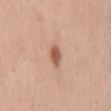Captured during whole-body skin photography for melanoma surveillance; the lesion was not biopsied. The total-body-photography lesion software estimated a footprint of about 4 mm², a shape eccentricity near 0.8, and a shape-asymmetry score of about 0.3 (0 = symmetric). The software also gave a mean CIELAB color near L≈57 a*≈23 b*≈31 and a normalized border contrast of about 8.5. The analysis additionally found a border-irregularity rating of about 2.5/10, a within-lesion color-variation index near 2.5/10, and a peripheral color-asymmetry measure near 1. It also reported a classifier nevus-likeness of about 95/100 and a detector confidence of about 100 out of 100 that the crop contains a lesion. A female patient, aged 33 to 37. The lesion's longest dimension is about 3 mm. A 15 mm close-up tile from a total-body photography series done for melanoma screening. On the mid back. This is a white-light tile.